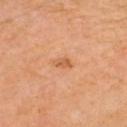No biopsy was performed on this lesion — it was imaged during a full skin examination and was not determined to be concerning. The tile uses cross-polarized illumination. The patient is a female aged 68 to 72. The lesion is on the head or neck. A region of skin cropped from a whole-body photographic capture, roughly 15 mm wide. The recorded lesion diameter is about 2 mm.